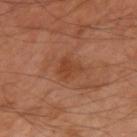biopsy status — total-body-photography surveillance lesion; no biopsy
site — the left upper arm
image source — 15 mm crop, total-body photography
subject — male, aged 48 to 52
illumination — cross-polarized
image-analysis metrics — a lesion area of about 4.5 mm² and an outline eccentricity of about 0.6 (0 = round, 1 = elongated); a mean CIELAB color near L≈41 a*≈25 b*≈33, a lesion–skin lightness drop of about 7, and a normalized lesion–skin contrast near 6; a border-irregularity rating of about 4.5/10
diameter — ~3 mm (longest diameter)